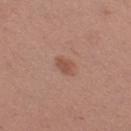Case summary:
– notes: no biopsy performed (imaged during a skin exam)
– diameter: ≈3 mm
– image-analysis metrics: an average lesion color of about L≈52 a*≈22 b*≈28 (CIELAB), about 8 CIELAB-L* units darker than the surrounding skin, and a normalized lesion–skin contrast near 6; a border-irregularity rating of about 2.5/10, internal color variation of about 1 on a 0–10 scale, and radial color variation of about 0.5; a lesion-detection confidence of about 100/100
– lighting: white-light illumination
– body site: the left thigh
– image source: ~15 mm tile from a whole-body skin photo
– subject: female, aged around 30